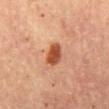The lesion was photographed on a routine skin check and not biopsied; there is no pathology result.
Located on the abdomen.
The lesion-visualizer software estimated a mean CIELAB color near L≈45 a*≈26 b*≈34 and a normalized border contrast of about 10.5. The analysis additionally found a within-lesion color-variation index near 3/10 and a peripheral color-asymmetry measure near 1. The analysis additionally found a nevus-likeness score of about 100/100.
Approximately 3 mm at its widest.
A female patient aged approximately 60.
This is a cross-polarized tile.
A 15 mm close-up tile from a total-body photography series done for melanoma screening.